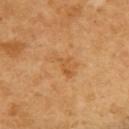Notes:
– notes: no biopsy performed (imaged during a skin exam)
– subject: female, aged around 55
– lesion size: ~4 mm (longest diameter)
– tile lighting: cross-polarized illumination
– location: the upper back
– acquisition: ~15 mm tile from a whole-body skin photo